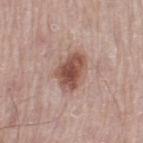The subject is a male aged 63–67. A close-up tile cropped from a whole-body skin photograph, about 15 mm across. From the leg.